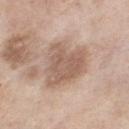Cropped from a total-body skin-imaging series; the visible field is about 15 mm.
A female patient about 55 years old.
The tile uses white-light illumination.
The lesion's longest dimension is about 5.5 mm.
On the left thigh.
Automated tile analysis of the lesion measured an average lesion color of about L≈59 a*≈17 b*≈27 (CIELAB) and a lesion-to-skin contrast of about 7 (normalized; higher = more distinct). The software also gave a color-variation rating of about 3/10 and radial color variation of about 1. The software also gave a classifier nevus-likeness of about 5/100 and a lesion-detection confidence of about 100/100.